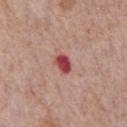Impression:
Part of a total-body skin-imaging series; this lesion was reviewed on a skin check and was not flagged for biopsy.
Acquisition and patient details:
Imaged with white-light lighting. An algorithmic analysis of the crop reported a lesion color around L≈47 a*≈35 b*≈24 in CIELAB, about 16 CIELAB-L* units darker than the surrounding skin, and a lesion-to-skin contrast of about 11 (normalized; higher = more distinct). Cropped from a total-body skin-imaging series; the visible field is about 15 mm. The patient is a male aged 58 to 62. Located on the chest. Approximately 2.5 mm at its widest.